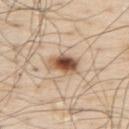Clinical impression: Part of a total-body skin-imaging series; this lesion was reviewed on a skin check and was not flagged for biopsy. Background: The lesion-visualizer software estimated a shape eccentricity near 0.85 and two-axis asymmetry of about 0.3. It also reported roughly 17 lightness units darker than nearby skin and a lesion-to-skin contrast of about 11 (normalized; higher = more distinct). The analysis additionally found border irregularity of about 3 on a 0–10 scale, a within-lesion color-variation index near 10/10, and radial color variation of about 3. And it measured a nevus-likeness score of about 100/100 and a lesion-detection confidence of about 100/100. A region of skin cropped from a whole-body photographic capture, roughly 15 mm wide. Imaged with white-light lighting. A male subject approximately 80 years of age. Located on the back.